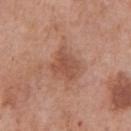subject: male, aged 68–72
imaging modality: total-body-photography crop, ~15 mm field of view
TBP lesion metrics: an outline eccentricity of about 0.6 (0 = round, 1 = elongated) and a symmetry-axis asymmetry near 0.3; a classifier nevus-likeness of about 0/100 and lesion-presence confidence of about 100/100
size: ~3.5 mm (longest diameter)
location: the front of the torso